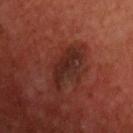{
  "biopsy_status": "not biopsied; imaged during a skin examination",
  "image": {
    "source": "total-body photography crop",
    "field_of_view_mm": 15
  },
  "patient": {
    "sex": "male",
    "age_approx": 60
  },
  "automated_metrics": {
    "area_mm2_approx": 8.5,
    "shape_asymmetry": 0.3,
    "cielab_L": 24,
    "cielab_a": 22,
    "cielab_b": 23,
    "vs_skin_darker_L": 8.0,
    "vs_skin_contrast_norm": 8.5,
    "nevus_likeness_0_100": 0,
    "lesion_detection_confidence_0_100": 95
  },
  "site": "chest"
}